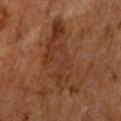Q: Was this lesion biopsied?
A: total-body-photography surveillance lesion; no biopsy
Q: How large is the lesion?
A: ~9.5 mm (longest diameter)
Q: Lesion location?
A: the arm
Q: What are the patient's age and sex?
A: male, about 65 years old
Q: What is the imaging modality?
A: 15 mm crop, total-body photography
Q: What lighting was used for the tile?
A: cross-polarized